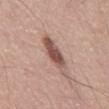Assessment: No biopsy was performed on this lesion — it was imaged during a full skin examination and was not determined to be concerning. Clinical summary: A male patient, aged 68–72. Imaged with white-light lighting. On the mid back. A 15 mm close-up tile from a total-body photography series done for melanoma screening. The total-body-photography lesion software estimated an average lesion color of about L≈53 a*≈20 b*≈25 (CIELAB), about 14 CIELAB-L* units darker than the surrounding skin, and a lesion-to-skin contrast of about 9 (normalized; higher = more distinct). Approximately 5 mm at its widest.